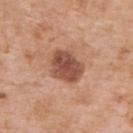Q: Was a biopsy performed?
A: no biopsy performed (imaged during a skin exam)
Q: What is the imaging modality?
A: total-body-photography crop, ~15 mm field of view
Q: Patient demographics?
A: male, approximately 60 years of age
Q: How large is the lesion?
A: about 4.5 mm
Q: Where on the body is the lesion?
A: the upper back
Q: What lighting was used for the tile?
A: white-light